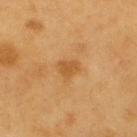The lesion was tiled from a total-body skin photograph and was not biopsied.
This image is a 15 mm lesion crop taken from a total-body photograph.
From the upper back.
The lesion-visualizer software estimated an area of roughly 4 mm², a shape eccentricity near 0.6, and a symmetry-axis asymmetry near 0.35. And it measured a border-irregularity index near 3/10 and internal color variation of about 1.5 on a 0–10 scale.
A male subject, aged around 60.